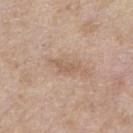biopsy_status: not biopsied; imaged during a skin examination
image:
  source: total-body photography crop
  field_of_view_mm: 15
automated_metrics:
  shape_asymmetry: 0.45
  vs_skin_darker_L: 8.0
  vs_skin_contrast_norm: 5.5
lesion_size:
  long_diameter_mm_approx: 4.0
site: arm
patient:
  sex: male
  age_approx: 60
lighting: white-light